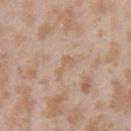Assessment: Imaged during a routine full-body skin examination; the lesion was not biopsied and no histopathology is available.